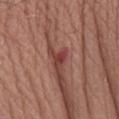Background:
Located on the left forearm. This image is a 15 mm lesion crop taken from a total-body photograph. Approximately 3 mm at its widest. This is a white-light tile. The subject is a male aged approximately 60.